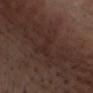| key | value |
|---|---|
| follow-up | imaged on a skin check; not biopsied |
| image | total-body-photography crop, ~15 mm field of view |
| anatomic site | the chest |
| subject | male, in their mid-70s |